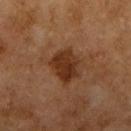No biopsy was performed on this lesion — it was imaged during a full skin examination and was not determined to be concerning.
This image is a 15 mm lesion crop taken from a total-body photograph.
Imaged with cross-polarized lighting.
Automated image analysis of the tile measured a lesion area of about 10 mm² and an outline eccentricity of about 0.65 (0 = round, 1 = elongated).
On the right upper arm.
The subject is a female approximately 60 years of age.